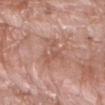{
  "biopsy_status": "not biopsied; imaged during a skin examination",
  "lighting": "white-light",
  "automated_metrics": {
    "eccentricity": 0.85,
    "shape_asymmetry": 0.65,
    "cielab_L": 56,
    "cielab_a": 22,
    "cielab_b": 28,
    "vs_skin_darker_L": 7.0,
    "vs_skin_contrast_norm": 5.0,
    "border_irregularity_0_10": 8.5,
    "color_variation_0_10": 4.0,
    "peripheral_color_asymmetry": 1.5
  },
  "patient": {
    "sex": "male",
    "age_approx": 60
  },
  "site": "right forearm",
  "image": {
    "source": "total-body photography crop",
    "field_of_view_mm": 15
  },
  "lesion_size": {
    "long_diameter_mm_approx": 5.5
  }
}